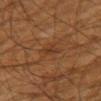Q: Was a biopsy performed?
A: imaged on a skin check; not biopsied
Q: Where on the body is the lesion?
A: the left forearm
Q: What is the lesion's diameter?
A: ≈2.5 mm
Q: How was this image acquired?
A: ~15 mm crop, total-body skin-cancer survey
Q: How was the tile lit?
A: cross-polarized illumination
Q: What are the patient's age and sex?
A: male, approximately 60 years of age